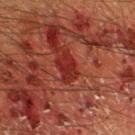Q: Was this lesion biopsied?
A: imaged on a skin check; not biopsied
Q: Who is the patient?
A: male, aged 63–67
Q: Lesion location?
A: the leg
Q: What is the imaging modality?
A: total-body-photography crop, ~15 mm field of view
Q: How large is the lesion?
A: about 3.5 mm
Q: What lighting was used for the tile?
A: cross-polarized